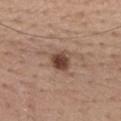<case>
  <lighting>white-light</lighting>
  <automated_metrics>
    <cielab_L>44</cielab_L>
    <cielab_a>19</cielab_a>
    <cielab_b>26</cielab_b>
    <vs_skin_darker_L>14.0</vs_skin_darker_L>
    <vs_skin_contrast_norm>10.5</vs_skin_contrast_norm>
    <border_irregularity_0_10>2.0</border_irregularity_0_10>
    <color_variation_0_10>5.0</color_variation_0_10>
    <peripheral_color_asymmetry>2.0</peripheral_color_asymmetry>
    <nevus_likeness_0_100>95</nevus_likeness_0_100>
    <lesion_detection_confidence_0_100>100</lesion_detection_confidence_0_100>
  </automated_metrics>
  <lesion_size>
    <long_diameter_mm_approx>2.5</long_diameter_mm_approx>
  </lesion_size>
  <patient>
    <sex>male</sex>
    <age_approx>55</age_approx>
  </patient>
  <image>
    <source>total-body photography crop</source>
    <field_of_view_mm>15</field_of_view_mm>
  </image>
  <site>mid back</site>
</case>